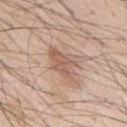The subject is a male aged 43–47. A region of skin cropped from a whole-body photographic capture, roughly 15 mm wide. About 5 mm across. On the back. The tile uses white-light illumination.